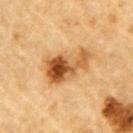Part of a total-body skin-imaging series; this lesion was reviewed on a skin check and was not flagged for biopsy. On the left upper arm. This is a cross-polarized tile. A male subject about 85 years old or older. Cropped from a total-body skin-imaging series; the visible field is about 15 mm.